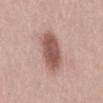{"biopsy_status": "not biopsied; imaged during a skin examination", "lesion_size": {"long_diameter_mm_approx": 6.0}, "image": {"source": "total-body photography crop", "field_of_view_mm": 15}, "patient": {"sex": "female", "age_approx": 60}, "automated_metrics": {"cielab_L": 55, "cielab_a": 21, "cielab_b": 24, "vs_skin_darker_L": 14.0}, "lighting": "white-light", "site": "mid back"}